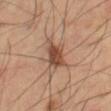Assessment: No biopsy was performed on this lesion — it was imaged during a full skin examination and was not determined to be concerning. Clinical summary: The patient is a male approximately 65 years of age. Longest diameter approximately 4 mm. A 15 mm close-up tile from a total-body photography series done for melanoma screening. The lesion is on the leg.